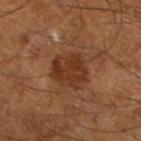Impression: Recorded during total-body skin imaging; not selected for excision or biopsy. Acquisition and patient details: A male patient, aged around 65. Approximately 4 mm at its widest. Imaged with cross-polarized lighting. The lesion is on the left lower leg. A roughly 15 mm field-of-view crop from a total-body skin photograph.